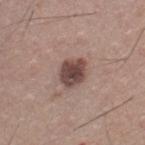No biopsy was performed on this lesion — it was imaged during a full skin examination and was not determined to be concerning. A male subject, aged around 40. An algorithmic analysis of the crop reported an area of roughly 7.5 mm², a shape eccentricity near 0.55, and two-axis asymmetry of about 0.15. The analysis additionally found a within-lesion color-variation index near 4/10 and peripheral color asymmetry of about 1. The analysis additionally found a classifier nevus-likeness of about 65/100. Approximately 3.5 mm at its widest. A 15 mm close-up tile from a total-body photography series done for melanoma screening. From the chest. The tile uses white-light illumination.